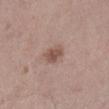biopsy status = catalogued during a skin exam; not biopsied
location = the right lower leg
subject = male, aged around 60
acquisition = 15 mm crop, total-body photography